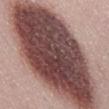This lesion was catalogued during total-body skin photography and was not selected for biopsy. The lesion is on the abdomen. A female patient approximately 50 years of age. Cropped from a total-body skin-imaging series; the visible field is about 15 mm.